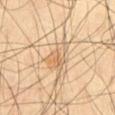<tbp_lesion>
  <biopsy_status>not biopsied; imaged during a skin examination</biopsy_status>
  <site>abdomen</site>
  <lesion_size>
    <long_diameter_mm_approx>4.5</long_diameter_mm_approx>
  </lesion_size>
  <image>
    <source>total-body photography crop</source>
    <field_of_view_mm>15</field_of_view_mm>
  </image>
  <lighting>cross-polarized</lighting>
  <automated_metrics>
    <cielab_L>65</cielab_L>
    <cielab_a>16</cielab_a>
    <cielab_b>36</cielab_b>
    <vs_skin_darker_L>8.0</vs_skin_darker_L>
    <vs_skin_contrast_norm>5.5</vs_skin_contrast_norm>
    <color_variation_0_10>5.5</color_variation_0_10>
    <peripheral_color_asymmetry>2.0</peripheral_color_asymmetry>
    <nevus_likeness_0_100>0</nevus_likeness_0_100>
    <lesion_detection_confidence_0_100>100</lesion_detection_confidence_0_100>
  </automated_metrics>
  <patient>
    <sex>male</sex>
    <age_approx>55</age_approx>
  </patient>
</tbp_lesion>